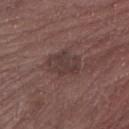Q: Was a biopsy performed?
A: no biopsy performed (imaged during a skin exam)
Q: What is the anatomic site?
A: the right forearm
Q: What lighting was used for the tile?
A: white-light illumination
Q: Lesion size?
A: ≈4 mm
Q: What is the imaging modality?
A: ~15 mm tile from a whole-body skin photo
Q: Who is the patient?
A: male, aged 68 to 72